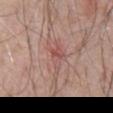Notes:
- workup · no biopsy performed (imaged during a skin exam)
- location · the abdomen
- patient · male, aged 58 to 62
- image · total-body-photography crop, ~15 mm field of view